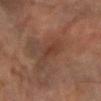A region of skin cropped from a whole-body photographic capture, roughly 15 mm wide. A male subject about 85 years old or older. On the left forearm. The recorded lesion diameter is about 2.5 mm. The tile uses cross-polarized illumination.